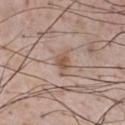| feature | finding |
|---|---|
| biopsy status | catalogued during a skin exam; not biopsied |
| image | ~15 mm crop, total-body skin-cancer survey |
| image-analysis metrics | a border-irregularity rating of about 4/10 |
| patient | male, aged around 55 |
| site | the front of the torso |
| lighting | white-light |